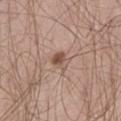| field | value |
|---|---|
| biopsy status | catalogued during a skin exam; not biopsied |
| lighting | white-light illumination |
| image | ~15 mm crop, total-body skin-cancer survey |
| diameter | ≈2.5 mm |
| subject | male, in their mid-60s |
| body site | the left thigh |